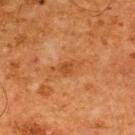| field | value |
|---|---|
| biopsy status | imaged on a skin check; not biopsied |
| size | ≈3.5 mm |
| acquisition | total-body-photography crop, ~15 mm field of view |
| patient | male, roughly 65 years of age |
| site | the upper back |
| tile lighting | cross-polarized |
| image-analysis metrics | a footprint of about 4 mm² and an outline eccentricity of about 0.85 (0 = round, 1 = elongated); a lesion color around L≈41 a*≈23 b*≈36 in CIELAB and a lesion-to-skin contrast of about 5.5 (normalized; higher = more distinct); a border-irregularity index near 4/10, a color-variation rating of about 1.5/10, and a peripheral color-asymmetry measure near 0.5; a lesion-detection confidence of about 100/100 |